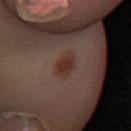workup: imaged on a skin check; not biopsied | illumination: cross-polarized illumination | subject: female, in their 50s | acquisition: total-body-photography crop, ~15 mm field of view | anatomic site: the left lower leg | size: ~3 mm (longest diameter) | image-analysis metrics: an area of roughly 6 mm², an outline eccentricity of about 0.6 (0 = round, 1 = elongated), and two-axis asymmetry of about 0.2; a lesion color around L≈27 a*≈15 b*≈19 in CIELAB; a classifier nevus-likeness of about 95/100 and a lesion-detection confidence of about 100/100.